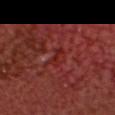workup = catalogued during a skin exam; not biopsied | lesion size = ~3 mm (longest diameter) | image source = ~15 mm crop, total-body skin-cancer survey | subject = male, aged 58 to 62 | body site = the head or neck | illumination = cross-polarized.